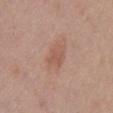Notes:
– workup · catalogued during a skin exam; not biopsied